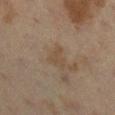Part of a total-body skin-imaging series; this lesion was reviewed on a skin check and was not flagged for biopsy.
A roughly 15 mm field-of-view crop from a total-body skin photograph.
The tile uses cross-polarized illumination.
A female patient, roughly 55 years of age.
The lesion is on the right lower leg.